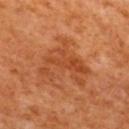Context: A 15 mm close-up extracted from a 3D total-body photography capture. The lesion-visualizer software estimated internal color variation of about 4 on a 0–10 scale and a peripheral color-asymmetry measure near 1.5. And it measured an automated nevus-likeness rating near 15 out of 100 and a lesion-detection confidence of about 100/100. A male subject, aged approximately 65. From the mid back. Measured at roughly 6.5 mm in maximum diameter. Imaged with cross-polarized lighting.